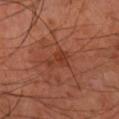No biopsy was performed on this lesion — it was imaged during a full skin examination and was not determined to be concerning.
Approximately 3 mm at its widest.
The subject is a male aged around 60.
An algorithmic analysis of the crop reported a lesion area of about 4.5 mm², a shape eccentricity near 0.75, and a shape-asymmetry score of about 0.55 (0 = symmetric). It also reported an average lesion color of about L≈33 a*≈25 b*≈29 (CIELAB), about 6 CIELAB-L* units darker than the surrounding skin, and a lesion-to-skin contrast of about 5.5 (normalized; higher = more distinct). And it measured a border-irregularity index near 6.5/10, a color-variation rating of about 2/10, and radial color variation of about 0.5.
The lesion is on the right forearm.
A lesion tile, about 15 mm wide, cut from a 3D total-body photograph.
The tile uses cross-polarized illumination.